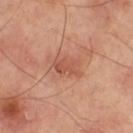biopsy status: total-body-photography surveillance lesion; no biopsy, imaging modality: ~15 mm tile from a whole-body skin photo, anatomic site: the right thigh.